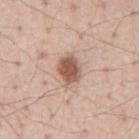Impression: Imaged during a routine full-body skin examination; the lesion was not biopsied and no histopathology is available. Context: The tile uses white-light illumination. A male patient aged 53–57. From the mid back. Longest diameter approximately 3.5 mm. A close-up tile cropped from a whole-body skin photograph, about 15 mm across. Automated tile analysis of the lesion measured a lesion area of about 7.5 mm², an eccentricity of roughly 0.7, and a shape-asymmetry score of about 0.2 (0 = symmetric). The software also gave a border-irregularity rating of about 2/10 and a peripheral color-asymmetry measure near 1.